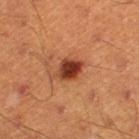Recorded during total-body skin imaging; not selected for excision or biopsy.
A roughly 15 mm field-of-view crop from a total-body skin photograph.
Imaged with cross-polarized lighting.
A male subject, approximately 60 years of age.
The total-body-photography lesion software estimated a footprint of about 6.5 mm² and two-axis asymmetry of about 0.2. The analysis additionally found a border-irregularity index near 2/10, a within-lesion color-variation index near 4.5/10, and radial color variation of about 1.5.
Measured at roughly 3.5 mm in maximum diameter.
On the right lower leg.